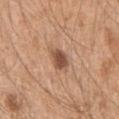Image and clinical context: On the left upper arm. A close-up tile cropped from a whole-body skin photograph, about 15 mm across. A male subject, aged 48 to 52. Measured at roughly 2.5 mm in maximum diameter.